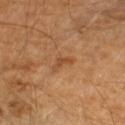biopsy_status: not biopsied; imaged during a skin examination
site: right upper arm
lighting: cross-polarized
automated_metrics:
  nevus_likeness_0_100: 0
  lesion_detection_confidence_0_100: 100
lesion_size:
  long_diameter_mm_approx: 3.0
patient:
  sex: male
  age_approx: 60
image:
  source: total-body photography crop
  field_of_view_mm: 15